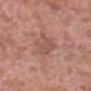follow-up = total-body-photography surveillance lesion; no biopsy
patient = male, aged approximately 50
lighting = white-light illumination
site = the head or neck
diameter = ≈4.5 mm
image source = ~15 mm tile from a whole-body skin photo